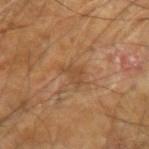This lesion was catalogued during total-body skin photography and was not selected for biopsy.
The patient is a male approximately 60 years of age.
A 15 mm crop from a total-body photograph taken for skin-cancer surveillance.
On the left arm.